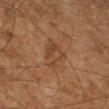Impression:
Captured during whole-body skin photography for melanoma surveillance; the lesion was not biopsied.
Clinical summary:
This image is a 15 mm lesion crop taken from a total-body photograph. On the left lower leg. A male patient, aged approximately 60.